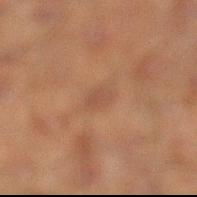Automated tile analysis of the lesion measured a footprint of about 2.5 mm², a shape eccentricity near 0.85, and two-axis asymmetry of about 0.25. The software also gave roughly 5 lightness units darker than nearby skin and a lesion-to-skin contrast of about 4.5 (normalized; higher = more distinct). And it measured a classifier nevus-likeness of about 0/100 and lesion-presence confidence of about 100/100. From the left lower leg. This image is a 15 mm lesion crop taken from a total-body photograph. About 2.5 mm across. A male subject, in their 50s. Captured under cross-polarized illumination.